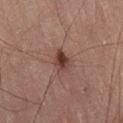biopsy_status: not biopsied; imaged during a skin examination
patient:
  sex: male
  age_approx: 50
automated_metrics:
  area_mm2_approx: 4.5
  eccentricity: 0.7
  shape_asymmetry: 0.2
  cielab_L: 35
  cielab_a: 18
  cielab_b: 21
  vs_skin_darker_L: 10.0
  vs_skin_contrast_norm: 9.0
  lesion_detection_confidence_0_100: 100
lighting: cross-polarized
image:
  source: total-body photography crop
  field_of_view_mm: 15
lesion_size:
  long_diameter_mm_approx: 3.0
site: right thigh